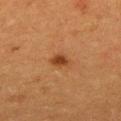  biopsy_status: not biopsied; imaged during a skin examination